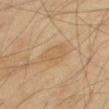Imaged during a routine full-body skin examination; the lesion was not biopsied and no histopathology is available.
The total-body-photography lesion software estimated a shape eccentricity near 0.85. The analysis additionally found an average lesion color of about L≈56 a*≈15 b*≈34 (CIELAB) and about 6 CIELAB-L* units darker than the surrounding skin.
A male patient, aged 68 to 72.
A roughly 15 mm field-of-view crop from a total-body skin photograph.
The lesion is on the right thigh.
The lesion's longest dimension is about 4.5 mm.